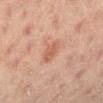Imaged with cross-polarized lighting. The patient is a female approximately 20 years of age. The total-body-photography lesion software estimated a footprint of about 4.5 mm² and a shape eccentricity near 0.7. It also reported a lesion color around L≈51 a*≈22 b*≈28 in CIELAB. The software also gave a detector confidence of about 100 out of 100 that the crop contains a lesion. On the right lower leg. A 15 mm close-up extracted from a 3D total-body photography capture. Longest diameter approximately 2.5 mm.